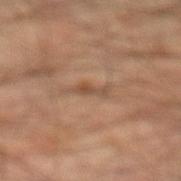Clinical impression:
Imaged during a routine full-body skin examination; the lesion was not biopsied and no histopathology is available.
Context:
About 3 mm across. From the leg. The subject is a male aged approximately 45. A lesion tile, about 15 mm wide, cut from a 3D total-body photograph. Automated tile analysis of the lesion measured an area of roughly 3 mm², a shape eccentricity near 0.95, and a symmetry-axis asymmetry near 0.4. It also reported a lesion color around L≈46 a*≈17 b*≈29 in CIELAB, about 8 CIELAB-L* units darker than the surrounding skin, and a normalized lesion–skin contrast near 6. It also reported a nevus-likeness score of about 0/100 and lesion-presence confidence of about 75/100. Captured under cross-polarized illumination.